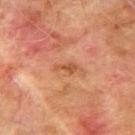Imaged during a routine full-body skin examination; the lesion was not biopsied and no histopathology is available.
The patient is a male aged 73–77.
On the arm.
A 15 mm crop from a total-body photograph taken for skin-cancer surveillance.
The recorded lesion diameter is about 2.5 mm.
The total-body-photography lesion software estimated an eccentricity of roughly 0.9 and a symmetry-axis asymmetry near 0.45. It also reported a border-irregularity rating of about 4.5/10 and radial color variation of about 0.5.